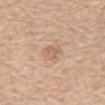<tbp_lesion>
<biopsy_status>not biopsied; imaged during a skin examination</biopsy_status>
<image>
  <source>total-body photography crop</source>
  <field_of_view_mm>15</field_of_view_mm>
</image>
<site>abdomen</site>
<lesion_size>
  <long_diameter_mm_approx>3.0</long_diameter_mm_approx>
</lesion_size>
<automated_metrics>
  <cielab_L>62</cielab_L>
  <cielab_a>19</cielab_a>
  <cielab_b>31</cielab_b>
  <color_variation_0_10>2.0</color_variation_0_10>
  <peripheral_color_asymmetry>0.5</peripheral_color_asymmetry>
  <nevus_likeness_0_100>0</nevus_likeness_0_100>
  <lesion_detection_confidence_0_100>100</lesion_detection_confidence_0_100>
</automated_metrics>
<lighting>white-light</lighting>
<patient>
  <sex>male</sex>
  <age_approx>70</age_approx>
</patient>
</tbp_lesion>